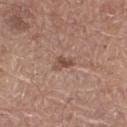notes: imaged on a skin check; not biopsied | diameter: ~2.5 mm (longest diameter) | lighting: white-light illumination | imaging modality: total-body-photography crop, ~15 mm field of view | patient: male, aged approximately 65 | TBP lesion metrics: a mean CIELAB color near L≈49 a*≈20 b*≈27, roughly 9 lightness units darker than nearby skin, and a lesion-to-skin contrast of about 7 (normalized; higher = more distinct); a border-irregularity rating of about 3.5/10 | anatomic site: the right thigh.